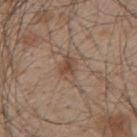Clinical impression:
No biopsy was performed on this lesion — it was imaged during a full skin examination and was not determined to be concerning.
Context:
A male patient, aged around 45. Longest diameter approximately 3.5 mm. The tile uses white-light illumination. A close-up tile cropped from a whole-body skin photograph, about 15 mm across. From the back.